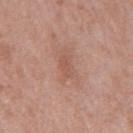follow-up: no biopsy performed (imaged during a skin exam) | site: the mid back | automated lesion analysis: an area of roughly 3 mm², an outline eccentricity of about 0.8 (0 = round, 1 = elongated), and two-axis asymmetry of about 0.3 | lesion size: ~2.5 mm (longest diameter) | acquisition: 15 mm crop, total-body photography | subject: male, in their 50s.